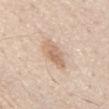The lesion's longest dimension is about 4.5 mm. A male subject, in their 80s. An algorithmic analysis of the crop reported about 11 CIELAB-L* units darker than the surrounding skin and a normalized lesion–skin contrast near 6.5. On the chest. A close-up tile cropped from a whole-body skin photograph, about 15 mm across.